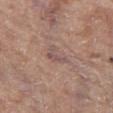The lesion was tiled from a total-body skin photograph and was not biopsied. The subject is a female roughly 75 years of age. About 3 mm across. From the right thigh. Cropped from a whole-body photographic skin survey; the tile spans about 15 mm.